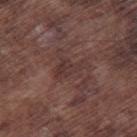The lesion was photographed on a routine skin check and not biopsied; there is no pathology result. Cropped from a whole-body photographic skin survey; the tile spans about 15 mm. The patient is a male aged around 75. The tile uses white-light illumination. The lesion is on the right thigh. Longest diameter approximately 4 mm. An algorithmic analysis of the crop reported a lesion area of about 6 mm², an outline eccentricity of about 0.85 (0 = round, 1 = elongated), and two-axis asymmetry of about 0.4. The software also gave a nevus-likeness score of about 0/100 and a lesion-detection confidence of about 70/100.